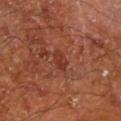Impression: Imaged during a routine full-body skin examination; the lesion was not biopsied and no histopathology is available. Clinical summary: A region of skin cropped from a whole-body photographic capture, roughly 15 mm wide. A male subject in their mid-60s. Located on the right lower leg. The tile uses cross-polarized illumination. An algorithmic analysis of the crop reported an area of roughly 3.5 mm², an eccentricity of roughly 0.8, and a shape-asymmetry score of about 0.3 (0 = symmetric). The analysis additionally found internal color variation of about 1.5 on a 0–10 scale. Longest diameter approximately 2.5 mm.